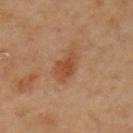{"biopsy_status": "not biopsied; imaged during a skin examination", "lighting": "cross-polarized", "lesion_size": {"long_diameter_mm_approx": 4.5}, "image": {"source": "total-body photography crop", "field_of_view_mm": 15}, "site": "left upper arm", "patient": {"sex": "female", "age_approx": 65}}